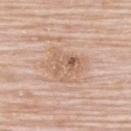follow-up: imaged on a skin check; not biopsied
image: 15 mm crop, total-body photography
image-analysis metrics: two-axis asymmetry of about 0.3; a lesion color around L≈62 a*≈18 b*≈30 in CIELAB, about 8 CIELAB-L* units darker than the surrounding skin, and a normalized border contrast of about 5.5; border irregularity of about 3 on a 0–10 scale and peripheral color asymmetry of about 2.5; an automated nevus-likeness rating near 0 out of 100
patient: female, aged approximately 65
anatomic site: the upper back
lesion size: about 4.5 mm